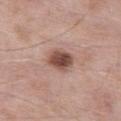notes — no biopsy performed (imaged during a skin exam)
patient — male, in their mid-50s
body site — the left thigh
acquisition — ~15 mm tile from a whole-body skin photo
lesion diameter — ≈3 mm
lighting — white-light